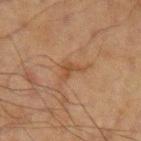Q: Is there a histopathology result?
A: imaged on a skin check; not biopsied
Q: What is the imaging modality?
A: ~15 mm crop, total-body skin-cancer survey
Q: Patient demographics?
A: male, aged approximately 70
Q: What did automated image analysis measure?
A: a footprint of about 3.5 mm² and an eccentricity of roughly 0.65; an automated nevus-likeness rating near 0 out of 100 and a detector confidence of about 100 out of 100 that the crop contains a lesion
Q: How was the tile lit?
A: cross-polarized
Q: How large is the lesion?
A: ≈3 mm
Q: What is the anatomic site?
A: the left upper arm